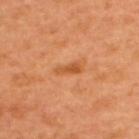This lesion was catalogued during total-body skin photography and was not selected for biopsy.